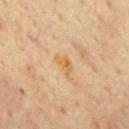No biopsy was performed on this lesion — it was imaged during a full skin examination and was not determined to be concerning. The lesion's longest dimension is about 3 mm. This is a cross-polarized tile. A close-up tile cropped from a whole-body skin photograph, about 15 mm across. The lesion is located on the mid back. A male patient, aged 63 to 67.